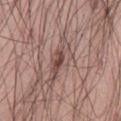Case summary:
* imaging modality · ~15 mm crop, total-body skin-cancer survey
* subject · male, aged 23–27
* location · the lower back
* TBP lesion metrics · a footprint of about 9 mm², a shape eccentricity near 0.95, and a shape-asymmetry score of about 0.45 (0 = symmetric); a within-lesion color-variation index near 7/10 and radial color variation of about 2
* size · about 6 mm